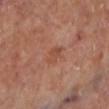Notes:
* follow-up — imaged on a skin check; not biopsied
* body site — the left lower leg
* image — ~15 mm crop, total-body skin-cancer survey
* diameter — ≈3 mm
* automated lesion analysis — a lesion color around L≈49 a*≈24 b*≈31 in CIELAB, about 6 CIELAB-L* units darker than the surrounding skin, and a lesion-to-skin contrast of about 5 (normalized; higher = more distinct)
* tile lighting — cross-polarized